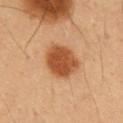The lesion was photographed on a routine skin check and not biopsied; there is no pathology result.
On the chest.
Cropped from a total-body skin-imaging series; the visible field is about 15 mm.
Captured under cross-polarized illumination.
Measured at roughly 4.5 mm in maximum diameter.
A male patient, aged 53–57.